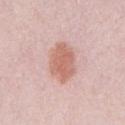Background:
The lesion is located on the chest. The subject is a male in their mid-20s. The tile uses white-light illumination. Automated tile analysis of the lesion measured a border-irregularity rating of about 2/10, internal color variation of about 3 on a 0–10 scale, and radial color variation of about 1. And it measured an automated nevus-likeness rating near 90 out of 100 and a lesion-detection confidence of about 100/100. A 15 mm close-up extracted from a 3D total-body photography capture.